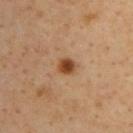  biopsy_status: not biopsied; imaged during a skin examination
  image:
    source: total-body photography crop
    field_of_view_mm: 15
  patient:
    sex: male
    age_approx: 65
  lighting: cross-polarized
  site: upper back
  automated_metrics:
    area_mm2_approx: 4.0
    shape_asymmetry: 0.25
    border_irregularity_0_10: 2.0
    peripheral_color_asymmetry: 0.5
  lesion_size:
    long_diameter_mm_approx: 2.0